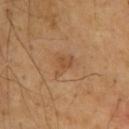This lesion was catalogued during total-body skin photography and was not selected for biopsy. The total-body-photography lesion software estimated a lesion area of about 4.5 mm², an eccentricity of roughly 0.75, and a symmetry-axis asymmetry near 0.5. Captured under cross-polarized illumination. A lesion tile, about 15 mm wide, cut from a 3D total-body photograph. Measured at roughly 3 mm in maximum diameter. A male subject aged approximately 65. Located on the back.